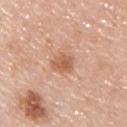A region of skin cropped from a whole-body photographic capture, roughly 15 mm wide.
On the chest.
A male patient, about 45 years old.